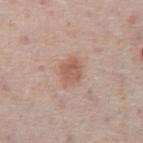<case>
<biopsy_status>not biopsied; imaged during a skin examination</biopsy_status>
<lesion_size>
  <long_diameter_mm_approx>2.5</long_diameter_mm_approx>
</lesion_size>
<site>abdomen</site>
<image>
  <source>total-body photography crop</source>
  <field_of_view_mm>15</field_of_view_mm>
</image>
<patient>
  <sex>male</sex>
  <age_approx>75</age_approx>
</patient>
<lighting>white-light</lighting>
</case>